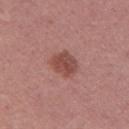Case summary:
* biopsy status · no biopsy performed (imaged during a skin exam)
* lesion size · ≈3 mm
* TBP lesion metrics · a shape eccentricity near 0.5 and two-axis asymmetry of about 0.15; a within-lesion color-variation index near 3.5/10 and a peripheral color-asymmetry measure near 1; a nevus-likeness score of about 75/100 and a detector confidence of about 100 out of 100 that the crop contains a lesion
* location · the right upper arm
* imaging modality · ~15 mm tile from a whole-body skin photo
* tile lighting · white-light illumination
* subject · female, approximately 50 years of age